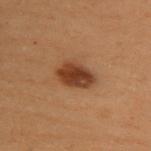workup: no biopsy performed (imaged during a skin exam)
lighting: cross-polarized
image-analysis metrics: an average lesion color of about L≈34 a*≈20 b*≈29 (CIELAB), roughly 13 lightness units darker than nearby skin, and a normalized border contrast of about 11; a border-irregularity index near 2/10 and a peripheral color-asymmetry measure near 1; a classifier nevus-likeness of about 100/100 and a detector confidence of about 100 out of 100 that the crop contains a lesion
diameter: ~4.5 mm (longest diameter)
body site: the upper back
imaging modality: total-body-photography crop, ~15 mm field of view
subject: female, in their 50s